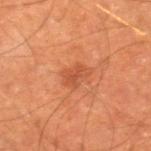From the right lower leg.
A 15 mm close-up extracted from a 3D total-body photography capture.
The lesion-visualizer software estimated a classifier nevus-likeness of about 25/100 and lesion-presence confidence of about 100/100.
A male patient aged 63 to 67.